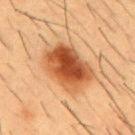notes: no biopsy performed (imaged during a skin exam)
anatomic site: the front of the torso
automated lesion analysis: a color-variation rating of about 8/10; a detector confidence of about 100 out of 100 that the crop contains a lesion
image source: ~15 mm tile from a whole-body skin photo
illumination: cross-polarized illumination
patient: male, in their mid- to late 50s